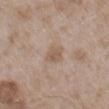The patient is a male aged approximately 50.
A close-up tile cropped from a whole-body skin photograph, about 15 mm across.
The lesion-visualizer software estimated a lesion–skin lightness drop of about 7 and a normalized lesion–skin contrast near 6.
Measured at roughly 2.5 mm in maximum diameter.
On the mid back.